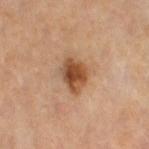A 15 mm crop from a total-body photograph taken for skin-cancer surveillance. A female patient roughly 50 years of age. Located on the right thigh. Captured under cross-polarized illumination. About 4 mm across.